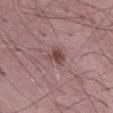Clinical impression:
Recorded during total-body skin imaging; not selected for excision or biopsy.
Background:
The lesion is on the front of the torso. Cropped from a whole-body photographic skin survey; the tile spans about 15 mm. A male patient, about 50 years old. Automated image analysis of the tile measured a lesion color around L≈46 a*≈21 b*≈21 in CIELAB, roughly 10 lightness units darker than nearby skin, and a lesion-to-skin contrast of about 8 (normalized; higher = more distinct). The software also gave border irregularity of about 3.5 on a 0–10 scale and a peripheral color-asymmetry measure near 0.5. It also reported an automated nevus-likeness rating near 80 out of 100 and a detector confidence of about 100 out of 100 that the crop contains a lesion. Imaged with white-light lighting. The lesion's longest dimension is about 2.5 mm.